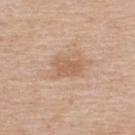Case summary:
• notes: total-body-photography surveillance lesion; no biopsy